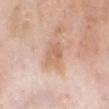Recorded during total-body skin imaging; not selected for excision or biopsy. A male patient approximately 70 years of age. This is a white-light tile. Located on the head or neck. Measured at roughly 3 mm in maximum diameter. A 15 mm close-up extracted from a 3D total-body photography capture.